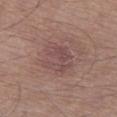Clinical impression:
Imaged during a routine full-body skin examination; the lesion was not biopsied and no histopathology is available.
Clinical summary:
The lesion is located on the left thigh. Cropped from a whole-body photographic skin survey; the tile spans about 15 mm. A male subject approximately 55 years of age. This is a white-light tile.